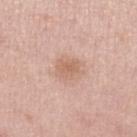{
  "biopsy_status": "not biopsied; imaged during a skin examination",
  "site": "leg",
  "lighting": "white-light",
  "image": {
    "source": "total-body photography crop",
    "field_of_view_mm": 15
  },
  "patient": {
    "sex": "female",
    "age_approx": 70
  }
}